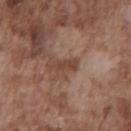Assessment: No biopsy was performed on this lesion — it was imaged during a full skin examination and was not determined to be concerning. Clinical summary: On the chest. A 15 mm close-up tile from a total-body photography series done for melanoma screening. This is a white-light tile. The recorded lesion diameter is about 3.5 mm. The total-body-photography lesion software estimated an average lesion color of about L≈43 a*≈19 b*≈27 (CIELAB), a lesion–skin lightness drop of about 8, and a lesion-to-skin contrast of about 7 (normalized; higher = more distinct). It also reported a border-irregularity rating of about 4.5/10, internal color variation of about 0.5 on a 0–10 scale, and a peripheral color-asymmetry measure near 0. A male subject aged approximately 75.